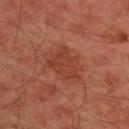The lesion was photographed on a routine skin check and not biopsied; there is no pathology result.
Automated image analysis of the tile measured a lesion color around L≈41 a*≈29 b*≈32 in CIELAB, a lesion–skin lightness drop of about 7, and a normalized border contrast of about 6. The analysis additionally found border irregularity of about 4 on a 0–10 scale, internal color variation of about 2.5 on a 0–10 scale, and a peripheral color-asymmetry measure near 1. The software also gave a lesion-detection confidence of about 100/100.
Located on the upper back.
Cropped from a total-body skin-imaging series; the visible field is about 15 mm.
A male subject, aged 43 to 47.